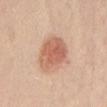Case summary:
• body site · the abdomen
• imaging modality · 15 mm crop, total-body photography
• image-analysis metrics · a footprint of about 14 mm² and an outline eccentricity of about 0.6 (0 = round, 1 = elongated); a lesion color around L≈62 a*≈24 b*≈31 in CIELAB, roughly 12 lightness units darker than nearby skin, and a lesion-to-skin contrast of about 7.5 (normalized; higher = more distinct); a classifier nevus-likeness of about 100/100 and a detector confidence of about 100 out of 100 that the crop contains a lesion
• patient · female, aged around 20
• illumination · white-light illumination